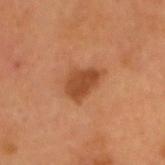follow-up: imaged on a skin check; not biopsied
acquisition: 15 mm crop, total-body photography
diameter: ~4 mm (longest diameter)
anatomic site: the head or neck
tile lighting: cross-polarized
patient: male, roughly 55 years of age
image-analysis metrics: a lesion area of about 8 mm², a shape eccentricity near 0.75, and a shape-asymmetry score of about 0.3 (0 = symmetric)